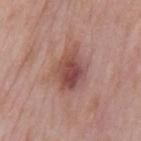Imaged during a routine full-body skin examination; the lesion was not biopsied and no histopathology is available.
Imaged with white-light lighting.
From the mid back.
The recorded lesion diameter is about 5.5 mm.
Cropped from a whole-body photographic skin survey; the tile spans about 15 mm.
A male subject, in their mid-50s.